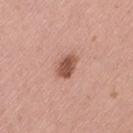Impression:
Part of a total-body skin-imaging series; this lesion was reviewed on a skin check and was not flagged for biopsy.
Acquisition and patient details:
A 15 mm close-up extracted from a 3D total-body photography capture. The total-body-photography lesion software estimated a footprint of about 5.5 mm², an eccentricity of roughly 0.75, and a shape-asymmetry score of about 0.2 (0 = symmetric). And it measured an average lesion color of about L≈53 a*≈24 b*≈28 (CIELAB), roughly 14 lightness units darker than nearby skin, and a normalized lesion–skin contrast near 9.5. The analysis additionally found a border-irregularity rating of about 2/10, internal color variation of about 2.5 on a 0–10 scale, and a peripheral color-asymmetry measure near 1. Located on the leg. Captured under white-light illumination. Measured at roughly 3 mm in maximum diameter. A female subject, roughly 60 years of age.